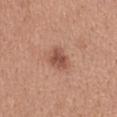Background: On the upper back. A female subject in their 30s. Cropped from a total-body skin-imaging series; the visible field is about 15 mm.